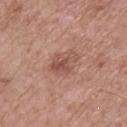lesion_size:
  long_diameter_mm_approx: 3.5
lighting: white-light
image:
  source: total-body photography crop
  field_of_view_mm: 15
site: upper back
patient:
  sex: male
  age_approx: 65